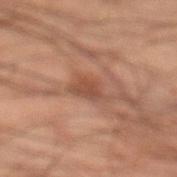The lesion was tiled from a total-body skin photograph and was not biopsied. Automated tile analysis of the lesion measured a classifier nevus-likeness of about 5/100 and a lesion-detection confidence of about 100/100. Located on the leg. Captured under cross-polarized illumination. A male patient, in their mid- to late 40s. Cropped from a total-body skin-imaging series; the visible field is about 15 mm. The lesion's longest dimension is about 4 mm.